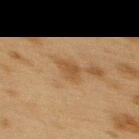  biopsy_status: not biopsied; imaged during a skin examination
  automated_metrics:
    cielab_L: 42
    cielab_a: 16
    cielab_b: 32
    vs_skin_contrast_norm: 6.0
    border_irregularity_0_10: 2.5
    color_variation_0_10: 1.5
    peripheral_color_asymmetry: 0.5
    nevus_likeness_0_100: 10
    lesion_detection_confidence_0_100: 100
  patient:
    sex: female
    age_approx: 40
  image:
    source: total-body photography crop
    field_of_view_mm: 15
  lighting: cross-polarized
  site: upper back
  lesion_size:
    long_diameter_mm_approx: 2.5